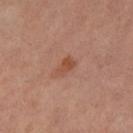Background:
This image is a 15 mm lesion crop taken from a total-body photograph. This is a cross-polarized tile. The lesion is on the left lower leg. A female subject, aged around 60. Automated image analysis of the tile measured roughly 8 lightness units darker than nearby skin and a normalized lesion–skin contrast near 7.